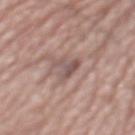The lesion was photographed on a routine skin check and not biopsied; there is no pathology result. The lesion is on the mid back. A male subject, in their mid- to late 70s. A roughly 15 mm field-of-view crop from a total-body skin photograph.